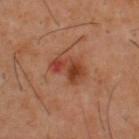Impression: Captured during whole-body skin photography for melanoma surveillance; the lesion was not biopsied. Acquisition and patient details: Longest diameter approximately 4.5 mm. A 15 mm close-up tile from a total-body photography series done for melanoma screening. A male subject, in their 40s. On the upper back.